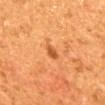The lesion was tiled from a total-body skin photograph and was not biopsied. Captured under cross-polarized illumination. A 15 mm close-up extracted from a 3D total-body photography capture. The subject is a male aged around 45. About 1.5 mm across. The lesion is on the mid back.